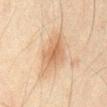workup: total-body-photography surveillance lesion; no biopsy
body site: the abdomen
patient: male, roughly 45 years of age
TBP lesion metrics: an outline eccentricity of about 0.8 (0 = round, 1 = elongated) and a symmetry-axis asymmetry near 0.4; a border-irregularity rating of about 4/10, a within-lesion color-variation index near 3.5/10, and a peripheral color-asymmetry measure near 1
tile lighting: cross-polarized illumination
acquisition: 15 mm crop, total-body photography